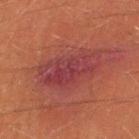{"biopsy_status": "not biopsied; imaged during a skin examination", "automated_metrics": {"area_mm2_approx": 21.0, "eccentricity": 0.85, "shape_asymmetry": 0.25, "nevus_likeness_0_100": 0, "lesion_detection_confidence_0_100": 95}, "site": "right thigh", "lighting": "cross-polarized", "image": {"source": "total-body photography crop", "field_of_view_mm": 15}, "patient": {"sex": "male", "age_approx": 40}, "lesion_size": {"long_diameter_mm_approx": 7.0}}